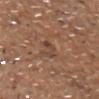biopsy status: imaged on a skin check; not biopsied
anatomic site: the front of the torso
tile lighting: white-light
subject: male, about 70 years old
size: ~3 mm (longest diameter)
imaging modality: ~15 mm crop, total-body skin-cancer survey